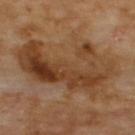Assessment:
The lesion was photographed on a routine skin check and not biopsied; there is no pathology result.
Image and clinical context:
The lesion is on the upper back. An algorithmic analysis of the crop reported an area of roughly 45 mm², an eccentricity of roughly 0.85, and a shape-asymmetry score of about 0.4 (0 = symmetric). The analysis additionally found a border-irregularity rating of about 6/10 and peripheral color asymmetry of about 3. The lesion's longest dimension is about 11 mm. The subject is a male in their 70s. Captured under cross-polarized illumination. Cropped from a total-body skin-imaging series; the visible field is about 15 mm.